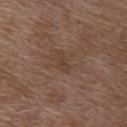This lesion was catalogued during total-body skin photography and was not selected for biopsy. A 15 mm close-up extracted from a 3D total-body photography capture. Located on the upper back. The subject is a female about 70 years old. An algorithmic analysis of the crop reported a lesion area of about 2.5 mm², an outline eccentricity of about 0.9 (0 = round, 1 = elongated), and a shape-asymmetry score of about 0.5 (0 = symmetric). The analysis additionally found an automated nevus-likeness rating near 0 out of 100 and a lesion-detection confidence of about 100/100. About 2.5 mm across.